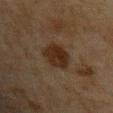– biopsy status · imaged on a skin check; not biopsied
– location · the upper back
– image source · ~15 mm tile from a whole-body skin photo
– patient · male, about 65 years old
– illumination · cross-polarized
– image-analysis metrics · an area of roughly 9.5 mm², an outline eccentricity of about 0.55 (0 = round, 1 = elongated), and two-axis asymmetry of about 0.15; border irregularity of about 1.5 on a 0–10 scale; a nevus-likeness score of about 70/100 and a lesion-detection confidence of about 100/100